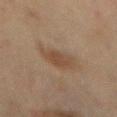<lesion>
  <biopsy_status>not biopsied; imaged during a skin examination</biopsy_status>
  <image>
    <source>total-body photography crop</source>
    <field_of_view_mm>15</field_of_view_mm>
  </image>
  <lesion_size>
    <long_diameter_mm_approx>4.0</long_diameter_mm_approx>
  </lesion_size>
  <lighting>cross-polarized</lighting>
  <automated_metrics>
    <area_mm2_approx>9.5</area_mm2_approx>
    <eccentricity>0.65</eccentricity>
    <shape_asymmetry>0.25</shape_asymmetry>
    <cielab_L>40</cielab_L>
    <cielab_a>14</cielab_a>
    <cielab_b>25</cielab_b>
    <vs_skin_darker_L>7.0</vs_skin_darker_L>
    <vs_skin_contrast_norm>6.0</vs_skin_contrast_norm>
    <peripheral_color_asymmetry>1.0</peripheral_color_asymmetry>
  </automated_metrics>
  <patient>
    <sex>male</sex>
    <age_approx>45</age_approx>
  </patient>
  <site>mid back</site>
</lesion>